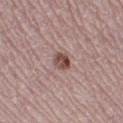workup — catalogued during a skin exam; not biopsied | patient — female, aged 48–52 | site — the right thigh | acquisition — 15 mm crop, total-body photography | lesion diameter — about 2.5 mm | automated metrics — an area of roughly 4 mm², an outline eccentricity of about 0.7 (0 = round, 1 = elongated), and a shape-asymmetry score of about 0.15 (0 = symmetric); a lesion color around L≈48 a*≈20 b*≈23 in CIELAB; a border-irregularity index near 1.5/10 and peripheral color asymmetry of about 2; a classifier nevus-likeness of about 85/100 and a lesion-detection confidence of about 100/100 | tile lighting — white-light illumination.